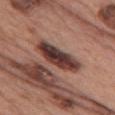notes: imaged on a skin check; not biopsied | image: ~15 mm crop, total-body skin-cancer survey | subject: female, approximately 60 years of age | site: the chest | lesion size: ≈6.5 mm | illumination: white-light.